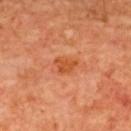Imaged during a routine full-body skin examination; the lesion was not biopsied and no histopathology is available.
The lesion-visualizer software estimated a nevus-likeness score of about 35/100.
The tile uses cross-polarized illumination.
A female subject in their 40s.
A region of skin cropped from a whole-body photographic capture, roughly 15 mm wide.
The lesion is on the upper back.
About 3 mm across.